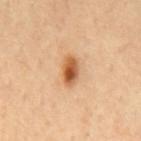Assessment: Captured during whole-body skin photography for melanoma surveillance; the lesion was not biopsied. Image and clinical context: This is a cross-polarized tile. A subject aged around 65. The lesion is located on the mid back. Automated tile analysis of the lesion measured a footprint of about 5.5 mm², a shape eccentricity near 0.85, and a symmetry-axis asymmetry near 0.25. The software also gave a lesion color around L≈57 a*≈24 b*≈40 in CIELAB and a lesion–skin lightness drop of about 15. Approximately 3.5 mm at its widest. Cropped from a whole-body photographic skin survey; the tile spans about 15 mm.